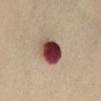Clinical impression: Recorded during total-body skin imaging; not selected for excision or biopsy. Context: The patient is a female aged around 55. A close-up tile cropped from a whole-body skin photograph, about 15 mm across. Located on the abdomen. Automated image analysis of the tile measured a lesion area of about 12 mm², an outline eccentricity of about 0.55 (0 = round, 1 = elongated), and two-axis asymmetry of about 0.15. And it measured about 24 CIELAB-L* units darker than the surrounding skin and a normalized border contrast of about 17. It also reported a border-irregularity rating of about 1.5/10, a within-lesion color-variation index near 10/10, and radial color variation of about 4. And it measured lesion-presence confidence of about 100/100.